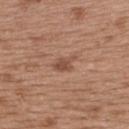Findings:
– image-analysis metrics: an area of roughly 4 mm²; border irregularity of about 4 on a 0–10 scale, a color-variation rating of about 1.5/10, and peripheral color asymmetry of about 0.5
– lighting: white-light
– site: the upper back
– image: ~15 mm tile from a whole-body skin photo
– diameter: about 3 mm
– subject: female, roughly 40 years of age